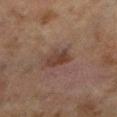Impression:
The lesion was photographed on a routine skin check and not biopsied; there is no pathology result.
Background:
Measured at roughly 3 mm in maximum diameter. On the leg. The subject is a female about 60 years old. Cropped from a whole-body photographic skin survey; the tile spans about 15 mm. Captured under cross-polarized illumination.